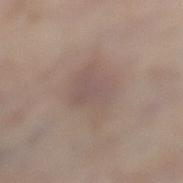Imaged during a routine full-body skin examination; the lesion was not biopsied and no histopathology is available.
A 15 mm close-up extracted from a 3D total-body photography capture.
Located on the left lower leg.
Automated image analysis of the tile measured an area of roughly 16 mm², a shape eccentricity near 0.7, and two-axis asymmetry of about 0.25. The software also gave a border-irregularity index near 3/10. The analysis additionally found a nevus-likeness score of about 0/100 and lesion-presence confidence of about 100/100.
A male patient aged 58–62.